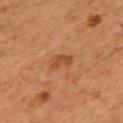Imaged during a routine full-body skin examination; the lesion was not biopsied and no histopathology is available.
This image is a 15 mm lesion crop taken from a total-body photograph.
Approximately 3 mm at its widest.
A male subject approximately 55 years of age.
From the mid back.
The tile uses cross-polarized illumination.